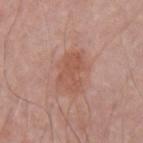Captured during whole-body skin photography for melanoma surveillance; the lesion was not biopsied. Cropped from a whole-body photographic skin survey; the tile spans about 15 mm. From the left upper arm. A male patient, aged 63–67. About 4.5 mm across.